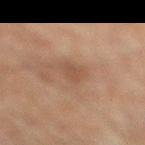Assessment:
Captured during whole-body skin photography for melanoma surveillance; the lesion was not biopsied.
Acquisition and patient details:
Captured under cross-polarized illumination. Longest diameter approximately 2.5 mm. Located on the right lower leg. Cropped from a whole-body photographic skin survey; the tile spans about 15 mm. A male subject approximately 65 years of age.